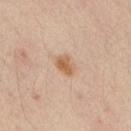biopsy_status: not biopsied; imaged during a skin examination
site: arm
patient:
  sex: male
  age_approx: 35
image:
  source: total-body photography crop
  field_of_view_mm: 15